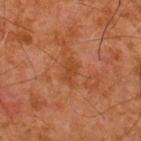Impression:
The lesion was tiled from a total-body skin photograph and was not biopsied.
Context:
The patient is a male approximately 65 years of age. A lesion tile, about 15 mm wide, cut from a 3D total-body photograph. On the upper back. The lesion's longest dimension is about 3 mm. Automated image analysis of the tile measured a lesion area of about 3.5 mm², an outline eccentricity of about 0.85 (0 = round, 1 = elongated), and a symmetry-axis asymmetry near 0.45. The analysis additionally found internal color variation of about 1 on a 0–10 scale and radial color variation of about 0.5.